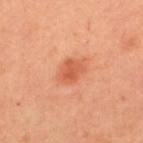{"site": "upper back", "image": {"source": "total-body photography crop", "field_of_view_mm": 15}, "lighting": "cross-polarized", "lesion_size": {"long_diameter_mm_approx": 3.0}, "patient": {"sex": "female", "age_approx": 45}}